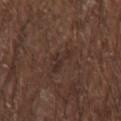Recorded during total-body skin imaging; not selected for excision or biopsy. Approximately 4 mm at its widest. The lesion is located on the arm. The subject is a male in their mid-60s. Imaged with white-light lighting. Cropped from a total-body skin-imaging series; the visible field is about 15 mm.